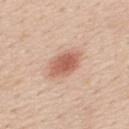This lesion was catalogued during total-body skin photography and was not selected for biopsy.
The lesion is on the mid back.
A male subject, aged 58–62.
The tile uses white-light illumination.
A 15 mm close-up tile from a total-body photography series done for melanoma screening.